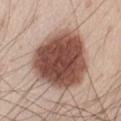Case summary:
* body site — the abdomen
* illumination — white-light
* image source — 15 mm crop, total-body photography
* subject — male, aged approximately 40
* diameter — about 7.5 mm